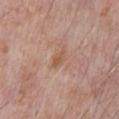The tile uses white-light illumination.
A 15 mm close-up tile from a total-body photography series done for melanoma screening.
The patient is a male in their 70s.
On the chest.
Automated tile analysis of the lesion measured an area of roughly 2.5 mm², a shape eccentricity near 0.85, and a shape-asymmetry score of about 0.2 (0 = symmetric). It also reported a border-irregularity rating of about 2.5/10, a color-variation rating of about 0.5/10, and peripheral color asymmetry of about 0. It also reported a nevus-likeness score of about 0/100 and a detector confidence of about 100 out of 100 that the crop contains a lesion.
Measured at roughly 2.5 mm in maximum diameter.